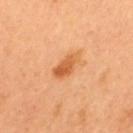Q: Is there a histopathology result?
A: imaged on a skin check; not biopsied
Q: How large is the lesion?
A: about 4 mm
Q: What kind of image is this?
A: ~15 mm tile from a whole-body skin photo
Q: How was the tile lit?
A: cross-polarized
Q: What are the patient's age and sex?
A: female, about 50 years old